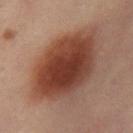Q: What kind of image is this?
A: ~15 mm tile from a whole-body skin photo
Q: Patient demographics?
A: female, aged around 40
Q: Lesion location?
A: the right thigh